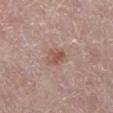{
  "biopsy_status": "not biopsied; imaged during a skin examination",
  "automated_metrics": {
    "area_mm2_approx": 5.0,
    "eccentricity": 0.75,
    "shape_asymmetry": 0.25,
    "vs_skin_darker_L": 9.0
  },
  "lesion_size": {
    "long_diameter_mm_approx": 3.0
  },
  "site": "leg",
  "image": {
    "source": "total-body photography crop",
    "field_of_view_mm": 15
  },
  "patient": {
    "sex": "female",
    "age_approx": 65
  },
  "lighting": "white-light"
}